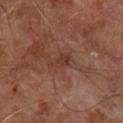follow-up: total-body-photography surveillance lesion; no biopsy | illumination: cross-polarized illumination | anatomic site: the right forearm | image: total-body-photography crop, ~15 mm field of view | patient: male, aged around 70 | diameter: ~3 mm (longest diameter).